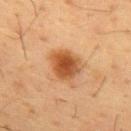This lesion was catalogued during total-body skin photography and was not selected for biopsy.
A 15 mm close-up extracted from a 3D total-body photography capture.
A male patient approximately 55 years of age.
The lesion is on the chest.
Imaged with cross-polarized lighting.